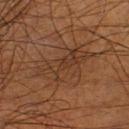Impression: The lesion was tiled from a total-body skin photograph and was not biopsied.